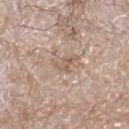Assessment:
The lesion was photographed on a routine skin check and not biopsied; there is no pathology result.
Context:
A male subject, aged 58–62. The lesion is on the right lower leg. Cropped from a whole-body photographic skin survey; the tile spans about 15 mm.